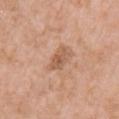Q: Was this lesion biopsied?
A: catalogued during a skin exam; not biopsied
Q: How was this image acquired?
A: total-body-photography crop, ~15 mm field of view
Q: What is the anatomic site?
A: the chest
Q: Patient demographics?
A: female, approximately 70 years of age